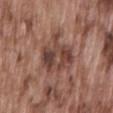{"biopsy_status": "not biopsied; imaged during a skin examination", "image": {"source": "total-body photography crop", "field_of_view_mm": 15}, "automated_metrics": {"cielab_L": 43, "cielab_a": 20, "cielab_b": 24, "vs_skin_darker_L": 10.0, "vs_skin_contrast_norm": 8.0, "border_irregularity_0_10": 4.0, "color_variation_0_10": 7.5, "peripheral_color_asymmetry": 2.5, "nevus_likeness_0_100": 10, "lesion_detection_confidence_0_100": 100}, "patient": {"sex": "male", "age_approx": 75}, "lesion_size": {"long_diameter_mm_approx": 5.0}, "lighting": "white-light", "site": "lower back"}